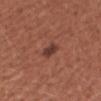Imaged during a routine full-body skin examination; the lesion was not biopsied and no histopathology is available. The lesion is located on the right forearm. The patient is a male aged 53 to 57. A 15 mm crop from a total-body photograph taken for skin-cancer surveillance.